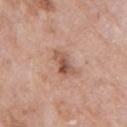Part of a total-body skin-imaging series; this lesion was reviewed on a skin check and was not flagged for biopsy. A female patient, aged 68–72. A 15 mm close-up extracted from a 3D total-body photography capture. Imaged with white-light lighting. From the arm.